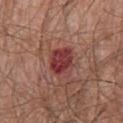Findings:
• follow-up — catalogued during a skin exam; not biopsied
• body site — the front of the torso
• automated metrics — roughly 12 lightness units darker than nearby skin; a nevus-likeness score of about 0/100 and lesion-presence confidence of about 100/100
• lesion diameter — ≈3.5 mm
• subject — male, aged around 65
• image — total-body-photography crop, ~15 mm field of view
• lighting — white-light